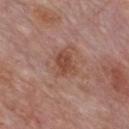Notes:
– notes · imaged on a skin check; not biopsied
– automated lesion analysis · a within-lesion color-variation index near 2.5/10 and a peripheral color-asymmetry measure near 1; lesion-presence confidence of about 100/100
– illumination · white-light
– lesion size · ~3.5 mm (longest diameter)
– acquisition · ~15 mm crop, total-body skin-cancer survey
– site · the chest
– subject · male, aged around 60